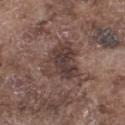Impression: Captured during whole-body skin photography for melanoma surveillance; the lesion was not biopsied. Acquisition and patient details: A region of skin cropped from a whole-body photographic capture, roughly 15 mm wide. The lesion is located on the left thigh. The lesion-visualizer software estimated a shape eccentricity near 0.5. A male subject, approximately 75 years of age. Longest diameter approximately 5.5 mm. This is a white-light tile.